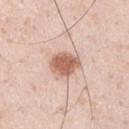– biopsy status: no biopsy performed (imaged during a skin exam)
– lighting: white-light
– location: the left upper arm
– patient: male, about 35 years old
– imaging modality: total-body-photography crop, ~15 mm field of view
– diameter: ≈3.5 mm